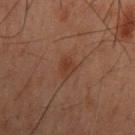Assessment:
The lesion was tiled from a total-body skin photograph and was not biopsied.
Clinical summary:
Approximately 2.5 mm at its widest. Automated image analysis of the tile measured a lesion color around L≈27 a*≈17 b*≈23 in CIELAB, roughly 5 lightness units darker than nearby skin, and a normalized border contrast of about 6. It also reported a border-irregularity rating of about 2/10, a within-lesion color-variation index near 2/10, and radial color variation of about 1. It also reported a classifier nevus-likeness of about 25/100 and lesion-presence confidence of about 100/100. The subject is a male roughly 60 years of age. This is a cross-polarized tile. Located on the mid back. A 15 mm crop from a total-body photograph taken for skin-cancer surveillance.